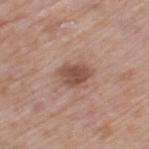Findings:
– acquisition — ~15 mm crop, total-body skin-cancer survey
– patient — male, aged around 80
– lesion size — ≈3.5 mm
– body site — the leg